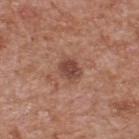Findings:
- workup — imaged on a skin check; not biopsied
- subject — male, aged approximately 65
- site — the upper back
- image-analysis metrics — an area of roughly 5.5 mm² and a symmetry-axis asymmetry near 0.2; a lesion color around L≈45 a*≈23 b*≈26 in CIELAB, about 11 CIELAB-L* units darker than the surrounding skin, and a normalized lesion–skin contrast near 8; a border-irregularity rating of about 2/10, a color-variation rating of about 4/10, and peripheral color asymmetry of about 1.5
- lesion diameter — ≈3 mm
- image — ~15 mm crop, total-body skin-cancer survey